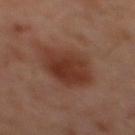Assessment:
The lesion was photographed on a routine skin check and not biopsied; there is no pathology result.
Image and clinical context:
This is a cross-polarized tile. A male patient, about 60 years old. The recorded lesion diameter is about 6.5 mm. A 15 mm close-up tile from a total-body photography series done for melanoma screening. The lesion is on the back. Automated tile analysis of the lesion measured an area of roughly 18 mm², an outline eccentricity of about 0.8 (0 = round, 1 = elongated), and two-axis asymmetry of about 0.2. And it measured border irregularity of about 2.5 on a 0–10 scale and peripheral color asymmetry of about 1.